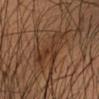biopsy_status: not biopsied; imaged during a skin examination